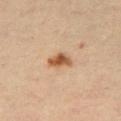notes=catalogued during a skin exam; not biopsied | lighting=cross-polarized | anatomic site=the right thigh | lesion diameter=≈3 mm | automated lesion analysis=a footprint of about 4.5 mm², an eccentricity of roughly 0.85, and a symmetry-axis asymmetry near 0.25; an automated nevus-likeness rating near 95 out of 100 and a detector confidence of about 100 out of 100 that the crop contains a lesion | imaging modality=15 mm crop, total-body photography | patient=female, aged 48–52.